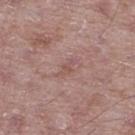Notes:
• tile lighting — white-light
• site — the right thigh
• image source — total-body-photography crop, ~15 mm field of view
• automated lesion analysis — an average lesion color of about L≈52 a*≈20 b*≈22 (CIELAB), about 6 CIELAB-L* units darker than the surrounding skin, and a normalized border contrast of about 4.5; a classifier nevus-likeness of about 0/100 and a detector confidence of about 100 out of 100 that the crop contains a lesion
• subject — male, aged 48–52
• diameter — ~2.5 mm (longest diameter)